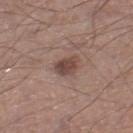The lesion was tiled from a total-body skin photograph and was not biopsied. A 15 mm close-up extracted from a 3D total-body photography capture. Located on the right lower leg. A male patient in their mid- to late 50s. The lesion's longest dimension is about 3 mm. Imaged with white-light lighting.